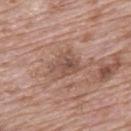| key | value |
|---|---|
| acquisition | ~15 mm crop, total-body skin-cancer survey |
| patient | male, aged around 70 |
| anatomic site | the upper back |
| lesion diameter | ~3.5 mm (longest diameter) |